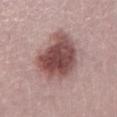Q: Was this lesion biopsied?
A: total-body-photography surveillance lesion; no biopsy
Q: Patient demographics?
A: male, aged around 30
Q: How was this image acquired?
A: ~15 mm crop, total-body skin-cancer survey